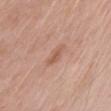This lesion was catalogued during total-body skin photography and was not selected for biopsy.
Longest diameter approximately 3 mm.
A male patient, in their mid-60s.
Located on the left upper arm.
The tile uses white-light illumination.
A lesion tile, about 15 mm wide, cut from a 3D total-body photograph.